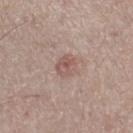Notes:
– workup — catalogued during a skin exam; not biopsied
– acquisition — total-body-photography crop, ~15 mm field of view
– site — the left thigh
– patient — male, aged around 65